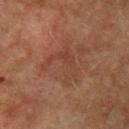Captured during whole-body skin photography for melanoma surveillance; the lesion was not biopsied.